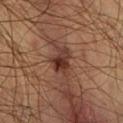Assessment:
No biopsy was performed on this lesion — it was imaged during a full skin examination and was not determined to be concerning.
Background:
Automated tile analysis of the lesion measured an area of roughly 7 mm², an outline eccentricity of about 0.65 (0 = round, 1 = elongated), and two-axis asymmetry of about 0.35. The analysis additionally found a lesion color around L≈32 a*≈20 b*≈24 in CIELAB, about 10 CIELAB-L* units darker than the surrounding skin, and a normalized border contrast of about 9.5. The software also gave border irregularity of about 4 on a 0–10 scale, a within-lesion color-variation index near 5.5/10, and a peripheral color-asymmetry measure near 2. And it measured a detector confidence of about 100 out of 100 that the crop contains a lesion. The tile uses cross-polarized illumination. A 15 mm crop from a total-body photograph taken for skin-cancer surveillance. The lesion is located on the chest. A male patient about 35 years old.